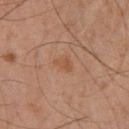* notes · no biopsy performed (imaged during a skin exam)
* image · ~15 mm crop, total-body skin-cancer survey
* illumination · cross-polarized illumination
* subject · male, aged 43–47
* body site · the right lower leg
* automated metrics · a detector confidence of about 100 out of 100 that the crop contains a lesion
* diameter · ≈2.5 mm